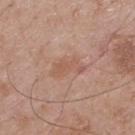location — the back; image-analysis metrics — a nevus-likeness score of about 0/100 and a lesion-detection confidence of about 100/100; illumination — white-light illumination; imaging modality — total-body-photography crop, ~15 mm field of view; subject — male, aged approximately 70; lesion size — about 4 mm.